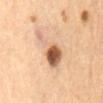Case summary:
• notes: catalogued during a skin exam; not biopsied
• subject: female, about 65 years old
• tile lighting: cross-polarized illumination
• site: the chest
• image-analysis metrics: a lesion area of about 11 mm², an eccentricity of roughly 0.8, and a symmetry-axis asymmetry near 0.55; a lesion color around L≈58 a*≈19 b*≈32 in CIELAB, about 15 CIELAB-L* units darker than the surrounding skin, and a normalized border contrast of about 9; a border-irregularity index near 6/10, a color-variation rating of about 9.5/10, and a peripheral color-asymmetry measure near 3; a classifier nevus-likeness of about 100/100 and a detector confidence of about 100 out of 100 that the crop contains a lesion
• image source: ~15 mm crop, total-body skin-cancer survey
• size: ≈6 mm